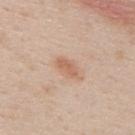Findings:
* workup — catalogued during a skin exam; not biopsied
* subject — male, in their 40s
* imaging modality — ~15 mm crop, total-body skin-cancer survey
* body site — the upper back
* size — ≈3 mm